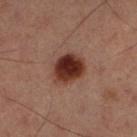{
  "biopsy_status": "not biopsied; imaged during a skin examination",
  "site": "left lower leg",
  "image": {
    "source": "total-body photography crop",
    "field_of_view_mm": 15
  },
  "patient": {
    "sex": "male",
    "age_approx": 60
  },
  "lesion_size": {
    "long_diameter_mm_approx": 4.0
  },
  "automated_metrics": {
    "area_mm2_approx": 10.0,
    "eccentricity": 0.6,
    "shape_asymmetry": 0.15,
    "cielab_L": 23,
    "cielab_a": 18,
    "cielab_b": 20,
    "vs_skin_darker_L": 13.0,
    "vs_skin_contrast_norm": 14.0,
    "color_variation_0_10": 4.0
  },
  "lighting": "cross-polarized"
}